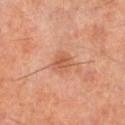A close-up tile cropped from a whole-body skin photograph, about 15 mm across.
The patient is a male about 60 years old.
On the left lower leg.